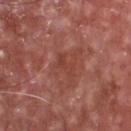Recorded during total-body skin imaging; not selected for excision or biopsy. About 3 mm across. The patient is a male roughly 65 years of age. A lesion tile, about 15 mm wide, cut from a 3D total-body photograph. Imaged with white-light lighting. Automated image analysis of the tile measured a footprint of about 3.5 mm², an eccentricity of roughly 0.9, and two-axis asymmetry of about 0.5. It also reported an automated nevus-likeness rating near 0 out of 100 and a detector confidence of about 100 out of 100 that the crop contains a lesion. On the chest.